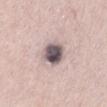Assessment:
The lesion was tiled from a total-body skin photograph and was not biopsied.
Clinical summary:
Measured at roughly 3 mm in maximum diameter. The tile uses white-light illumination. Located on the right upper arm. Cropped from a total-body skin-imaging series; the visible field is about 15 mm. Automated image analysis of the tile measured a footprint of about 7.5 mm² and a symmetry-axis asymmetry near 0.15. The analysis additionally found a mean CIELAB color near L≈52 a*≈13 b*≈12, about 21 CIELAB-L* units darker than the surrounding skin, and a normalized lesion–skin contrast near 14. And it measured a border-irregularity index near 1.5/10, a within-lesion color-variation index near 7/10, and peripheral color asymmetry of about 2.5. The patient is a male roughly 65 years of age.